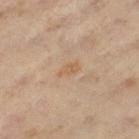Notes:
* notes · imaged on a skin check; not biopsied
* patient · male, aged 58 to 62
* TBP lesion metrics · a lesion area of about 3 mm², an outline eccentricity of about 0.85 (0 = round, 1 = elongated), and two-axis asymmetry of about 0.3; a border-irregularity index near 3.5/10, a color-variation rating of about 1.5/10, and radial color variation of about 0.5; an automated nevus-likeness rating near 0 out of 100
* image · total-body-photography crop, ~15 mm field of view
* lesion size · about 3 mm
* tile lighting · cross-polarized
* location · the left thigh